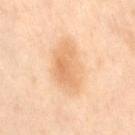site: right thigh
lighting: cross-polarized
automated_metrics:
  cielab_L: 71
  cielab_a: 20
  cielab_b: 39
  vs_skin_darker_L: 9.0
  vs_skin_contrast_norm: 6.0
  border_irregularity_0_10: 2.0
  color_variation_0_10: 3.5
  peripheral_color_asymmetry: 1.0
lesion_size:
  long_diameter_mm_approx: 6.0
patient:
  sex: female
  age_approx: 50
image:
  source: total-body photography crop
  field_of_view_mm: 15